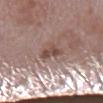workup: imaged on a skin check; not biopsied | acquisition: ~15 mm crop, total-body skin-cancer survey | lesion diameter: ≈2.5 mm | site: the leg | patient: female, aged approximately 70 | lighting: white-light illumination | automated metrics: a lesion color around L≈48 a*≈18 b*≈22 in CIELAB, roughly 9 lightness units darker than nearby skin, and a lesion-to-skin contrast of about 7 (normalized; higher = more distinct).